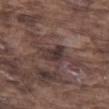follow-up: catalogued during a skin exam; not biopsied
patient: male, aged 73 to 77
anatomic site: the leg
diameter: ~3 mm (longest diameter)
image-analysis metrics: an area of roughly 5 mm², an outline eccentricity of about 0.65 (0 = round, 1 = elongated), and two-axis asymmetry of about 0.4
acquisition: 15 mm crop, total-body photography